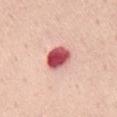Recorded during total-body skin imaging; not selected for excision or biopsy.
A male patient, aged approximately 50.
The lesion is on the mid back.
Automated image analysis of the tile measured an outline eccentricity of about 0.25 (0 = round, 1 = elongated). It also reported a mean CIELAB color near L≈55 a*≈37 b*≈24. And it measured a color-variation rating of about 6.5/10 and radial color variation of about 2.
Imaged with white-light lighting.
Longest diameter approximately 3 mm.
A 15 mm close-up extracted from a 3D total-body photography capture.